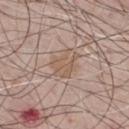Clinical impression: Recorded during total-body skin imaging; not selected for excision or biopsy. Image and clinical context: The lesion is on the chest. A 15 mm close-up tile from a total-body photography series done for melanoma screening. Approximately 4 mm at its widest. A male subject, aged 63–67.